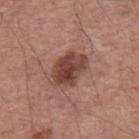Imaged during a routine full-body skin examination; the lesion was not biopsied and no histopathology is available.
The patient is a male aged 53 to 57.
This is a white-light tile.
The recorded lesion diameter is about 4.5 mm.
A 15 mm close-up extracted from a 3D total-body photography capture.
The lesion is located on the mid back.